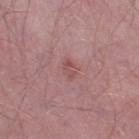This lesion was catalogued during total-body skin photography and was not selected for biopsy. This is a white-light tile. A close-up tile cropped from a whole-body skin photograph, about 15 mm across. A male subject, aged around 55. Automated tile analysis of the lesion measured an eccentricity of roughly 0.75 and a shape-asymmetry score of about 0.25 (0 = symmetric). It also reported a border-irregularity index near 2/10, a within-lesion color-variation index near 2/10, and radial color variation of about 1. And it measured a lesion-detection confidence of about 100/100. Located on the right thigh.